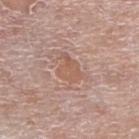Case summary:
– biopsy status · catalogued during a skin exam; not biopsied
– image · ~15 mm tile from a whole-body skin photo
– location · the leg
– patient · male, roughly 80 years of age